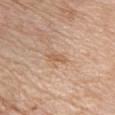The lesion was photographed on a routine skin check and not biopsied; there is no pathology result. On the chest. A region of skin cropped from a whole-body photographic capture, roughly 15 mm wide. The recorded lesion diameter is about 2.5 mm. Automated tile analysis of the lesion measured a lesion color around L≈59 a*≈19 b*≈33 in CIELAB, roughly 8 lightness units darker than nearby skin, and a lesion-to-skin contrast of about 6 (normalized; higher = more distinct). The analysis additionally found a border-irregularity index near 3.5/10, a within-lesion color-variation index near 0/10, and a peripheral color-asymmetry measure near 0. Captured under white-light illumination. The subject is a female aged around 70.